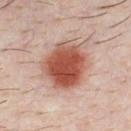<lesion>
<patient>
  <sex>male</sex>
  <age_approx>30</age_approx>
</patient>
<lighting>cross-polarized</lighting>
<site>chest</site>
<image>
  <source>total-body photography crop</source>
  <field_of_view_mm>15</field_of_view_mm>
</image>
<diagnosis>
  <histopathology>compound melanocytic nevus</histopathology>
  <malignancy>benign</malignancy>
  <taxonomic_path>Benign; Benign melanocytic proliferations; Nevus; Nevus, NOS, Compound</taxonomic_path>
</diagnosis>
</lesion>